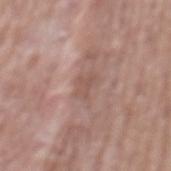Q: Was this lesion biopsied?
A: imaged on a skin check; not biopsied
Q: Automated lesion metrics?
A: a mean CIELAB color near L≈53 a*≈19 b*≈24, about 7 CIELAB-L* units darker than the surrounding skin, and a normalized border contrast of about 5.5
Q: What lighting was used for the tile?
A: white-light
Q: What are the patient's age and sex?
A: male, approximately 75 years of age
Q: What is the imaging modality?
A: ~15 mm crop, total-body skin-cancer survey
Q: Lesion location?
A: the mid back
Q: What is the lesion's diameter?
A: ≈2.5 mm